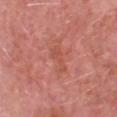follow-up — no biopsy performed (imaged during a skin exam)
anatomic site — the head or neck
subject — male, in their 70s
image — 15 mm crop, total-body photography